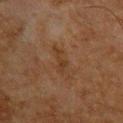Q: Was this lesion biopsied?
A: imaged on a skin check; not biopsied
Q: Automated lesion metrics?
A: a lesion color around L≈29 a*≈16 b*≈27 in CIELAB, roughly 5 lightness units darker than nearby skin, and a normalized lesion–skin contrast near 5.5; a detector confidence of about 100 out of 100 that the crop contains a lesion
Q: Illumination type?
A: cross-polarized
Q: How large is the lesion?
A: ≈3 mm
Q: What kind of image is this?
A: ~15 mm tile from a whole-body skin photo
Q: Who is the patient?
A: male, about 65 years old
Q: What is the anatomic site?
A: the upper back